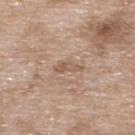{"biopsy_status": "not biopsied; imaged during a skin examination", "image": {"source": "total-body photography crop", "field_of_view_mm": 15}, "lesion_size": {"long_diameter_mm_approx": 3.5}, "site": "upper back", "patient": {"sex": "female", "age_approx": 75}}